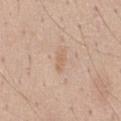Clinical impression:
Imaged during a routine full-body skin examination; the lesion was not biopsied and no histopathology is available.
Context:
The lesion-visualizer software estimated an area of roughly 3 mm², an eccentricity of roughly 0.9, and a symmetry-axis asymmetry near 0.35. Approximately 3 mm at its widest. A male subject, aged 38–42. The lesion is located on the chest. A 15 mm close-up extracted from a 3D total-body photography capture. Captured under white-light illumination.